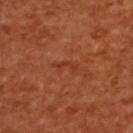Case summary:
• biopsy status · no biopsy performed (imaged during a skin exam)
• automated metrics · a border-irregularity index near 7/10, a color-variation rating of about 0/10, and a peripheral color-asymmetry measure near 0; an automated nevus-likeness rating near 0 out of 100
• subject · female, aged 53 to 57
• site · the back
• tile lighting · cross-polarized illumination
• acquisition · ~15 mm crop, total-body skin-cancer survey
• size · about 2.5 mm